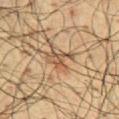This lesion was catalogued during total-body skin photography and was not selected for biopsy. The lesion is located on the chest. A 15 mm close-up extracted from a 3D total-body photography capture. An algorithmic analysis of the crop reported a classifier nevus-likeness of about 5/100. The patient is a male roughly 60 years of age. About 3.5 mm across. This is a cross-polarized tile.